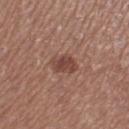Q: What kind of image is this?
A: ~15 mm crop, total-body skin-cancer survey
Q: What are the patient's age and sex?
A: female, aged 43–47
Q: Illumination type?
A: white-light illumination
Q: What is the anatomic site?
A: the right lower leg
Q: What is the lesion's diameter?
A: ≈3.5 mm